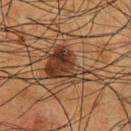biopsy status = catalogued during a skin exam; not biopsied
patient = male, aged 53 to 57
tile lighting = cross-polarized illumination
automated lesion analysis = an area of roughly 16 mm², an eccentricity of roughly 0.8, and two-axis asymmetry of about 0.5; a normalized lesion–skin contrast near 11; a peripheral color-asymmetry measure near 2.5
image source = ~15 mm tile from a whole-body skin photo
diameter = ≈6.5 mm
location = the chest